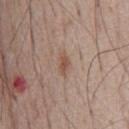follow-up=catalogued during a skin exam; not biopsied | size=≈2.5 mm | patient=male, approximately 55 years of age | automated lesion analysis=a footprint of about 2.5 mm² and a symmetry-axis asymmetry near 0.3 | image=~15 mm tile from a whole-body skin photo | lighting=white-light illumination.